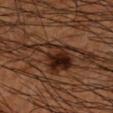{"biopsy_status": "not biopsied; imaged during a skin examination", "lesion_size": {"long_diameter_mm_approx": 6.0}, "site": "right forearm", "patient": {"sex": "male", "age_approx": 65}, "image": {"source": "total-body photography crop", "field_of_view_mm": 15}}